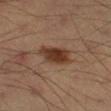workup = catalogued during a skin exam; not biopsied
size = about 4 mm
illumination = cross-polarized
image source = ~15 mm tile from a whole-body skin photo
site = the right lower leg
patient = male, aged 63–67